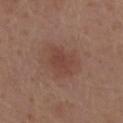biopsy status: total-body-photography surveillance lesion; no biopsy | size: about 3.5 mm | location: the upper back | image source: total-body-photography crop, ~15 mm field of view | subject: male, aged approximately 70 | tile lighting: white-light illumination | TBP lesion metrics: a lesion-to-skin contrast of about 5.5 (normalized; higher = more distinct); border irregularity of about 2.5 on a 0–10 scale, a color-variation rating of about 2/10, and a peripheral color-asymmetry measure near 0.5.